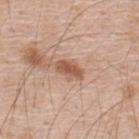Q: Was this lesion biopsied?
A: catalogued during a skin exam; not biopsied
Q: What is the lesion's diameter?
A: about 3.5 mm
Q: Who is the patient?
A: male, aged around 50
Q: Where on the body is the lesion?
A: the upper back
Q: How was the tile lit?
A: white-light
Q: What is the imaging modality?
A: ~15 mm crop, total-body skin-cancer survey